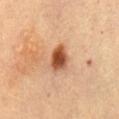{
  "lighting": "cross-polarized",
  "lesion_size": {
    "long_diameter_mm_approx": 3.5
  },
  "site": "abdomen",
  "patient": {
    "sex": "female",
    "age_approx": 60
  },
  "image": {
    "source": "total-body photography crop",
    "field_of_view_mm": 15
  }
}